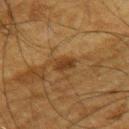Clinical impression: Captured during whole-body skin photography for melanoma surveillance; the lesion was not biopsied. Image and clinical context: The lesion is located on the upper back. Automated tile analysis of the lesion measured an eccentricity of roughly 0.85 and a symmetry-axis asymmetry near 0.2. And it measured a lesion color around L≈31 a*≈16 b*≈30 in CIELAB and about 8 CIELAB-L* units darker than the surrounding skin. The analysis additionally found a border-irregularity rating of about 2.5/10, a color-variation rating of about 1.5/10, and radial color variation of about 0.5. And it measured an automated nevus-likeness rating near 30 out of 100 and a lesion-detection confidence of about 95/100. This is a cross-polarized tile. A male patient about 65 years old. A region of skin cropped from a whole-body photographic capture, roughly 15 mm wide.